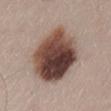The lesion was photographed on a routine skin check and not biopsied; there is no pathology result.
Automated image analysis of the tile measured a lesion area of about 40 mm², a shape eccentricity near 0.75, and a symmetry-axis asymmetry near 0.15. It also reported a border-irregularity index near 2/10, a within-lesion color-variation index near 10/10, and radial color variation of about 4.5. The software also gave a classifier nevus-likeness of about 100/100 and a detector confidence of about 100 out of 100 that the crop contains a lesion.
A 15 mm crop from a total-body photograph taken for skin-cancer surveillance.
A male subject, aged 53–57.
The lesion is on the lower back.